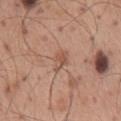Q: Was this lesion biopsied?
A: catalogued during a skin exam; not biopsied
Q: How large is the lesion?
A: about 3 mm
Q: How was this image acquired?
A: ~15 mm crop, total-body skin-cancer survey
Q: What are the patient's age and sex?
A: male, aged around 60
Q: Where on the body is the lesion?
A: the mid back
Q: Automated lesion metrics?
A: a shape eccentricity near 0.8 and a shape-asymmetry score of about 0.35 (0 = symmetric); roughly 8 lightness units darker than nearby skin and a lesion-to-skin contrast of about 6 (normalized; higher = more distinct); border irregularity of about 3.5 on a 0–10 scale, a within-lesion color-variation index near 1.5/10, and a peripheral color-asymmetry measure near 0.5
Q: How was the tile lit?
A: white-light